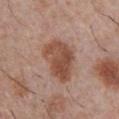Captured during whole-body skin photography for melanoma surveillance; the lesion was not biopsied. A male patient, approximately 30 years of age. A roughly 15 mm field-of-view crop from a total-body skin photograph. Imaged with white-light lighting. The lesion is on the chest.